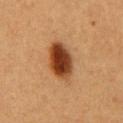Part of a total-body skin-imaging series; this lesion was reviewed on a skin check and was not flagged for biopsy.
The recorded lesion diameter is about 5.5 mm.
Automated tile analysis of the lesion measured a mean CIELAB color near L≈40 a*≈23 b*≈35 and a normalized lesion–skin contrast near 13. The analysis additionally found an automated nevus-likeness rating near 100 out of 100 and a lesion-detection confidence of about 100/100.
Imaged with cross-polarized lighting.
A roughly 15 mm field-of-view crop from a total-body skin photograph.
A male subject, approximately 60 years of age.
The lesion is on the chest.